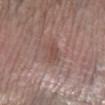site=the right lower leg
subject=female, aged 83 or older
image=total-body-photography crop, ~15 mm field of view
illumination=white-light illumination
automated lesion analysis=border irregularity of about 2.5 on a 0–10 scale, a within-lesion color-variation index near 1.5/10, and a peripheral color-asymmetry measure near 0.5; a nevus-likeness score of about 0/100
size=≈3 mm